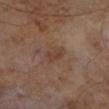Notes:
• diameter · about 3 mm
• tile lighting · cross-polarized
• subject · male, aged 63 to 67
• image source · ~15 mm tile from a whole-body skin photo